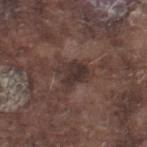This lesion was catalogued during total-body skin photography and was not selected for biopsy.
The subject is a male aged around 75.
Located on the right lower leg.
A region of skin cropped from a whole-body photographic capture, roughly 15 mm wide.